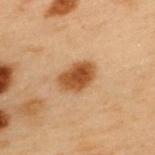Imaged during a routine full-body skin examination; the lesion was not biopsied and no histopathology is available. The total-body-photography lesion software estimated an area of roughly 8.5 mm² and a shape eccentricity near 0.75. The software also gave a within-lesion color-variation index near 3.5/10 and a peripheral color-asymmetry measure near 1. It also reported a classifier nevus-likeness of about 100/100 and lesion-presence confidence of about 100/100. The lesion is on the upper back. A roughly 15 mm field-of-view crop from a total-body skin photograph. A male subject approximately 55 years of age. About 4 mm across.